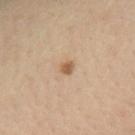<record>
<biopsy_status>not biopsied; imaged during a skin examination</biopsy_status>
<patient>
  <sex>female</sex>
  <age_approx>40</age_approx>
</patient>
<site>arm</site>
<image>
  <source>total-body photography crop</source>
  <field_of_view_mm>15</field_of_view_mm>
</image>
</record>